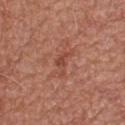workup: no biopsy performed (imaged during a skin exam) | site: the upper back | automated lesion analysis: a footprint of about 4 mm² and an eccentricity of roughly 0.85; a mean CIELAB color near L≈47 a*≈26 b*≈30, roughly 7 lightness units darker than nearby skin, and a lesion-to-skin contrast of about 5.5 (normalized; higher = more distinct); a nevus-likeness score of about 0/100 | lighting: white-light | image source: 15 mm crop, total-body photography | patient: male, approximately 65 years of age | lesion size: ~3.5 mm (longest diameter).